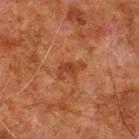The lesion was tiled from a total-body skin photograph and was not biopsied.
An algorithmic analysis of the crop reported a border-irregularity index near 4/10 and a color-variation rating of about 1.5/10. The analysis additionally found an automated nevus-likeness rating near 5 out of 100 and a lesion-detection confidence of about 100/100.
Located on the upper back.
This image is a 15 mm lesion crop taken from a total-body photograph.
The subject is a male aged approximately 80.